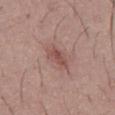{
  "biopsy_status": "not biopsied; imaged during a skin examination",
  "image": {
    "source": "total-body photography crop",
    "field_of_view_mm": 15
  },
  "lighting": "white-light",
  "site": "abdomen",
  "automated_metrics": {
    "border_irregularity_0_10": 2.0,
    "color_variation_0_10": 3.5,
    "peripheral_color_asymmetry": 1.0,
    "nevus_likeness_0_100": 5
  },
  "patient": {
    "sex": "male",
    "age_approx": 45
  }
}